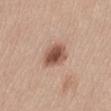Clinical impression: This lesion was catalogued during total-body skin photography and was not selected for biopsy. Background: Approximately 3.5 mm at its widest. On the abdomen. A 15 mm close-up extracted from a 3D total-body photography capture. A female patient in their mid-20s. The tile uses white-light illumination.